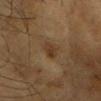– notes — catalogued during a skin exam; not biopsied
– anatomic site — the head or neck
– imaging modality — 15 mm crop, total-body photography
– lighting — cross-polarized illumination
– patient — female, aged around 60
– diameter — ~3 mm (longest diameter)